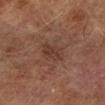Impression:
Recorded during total-body skin imaging; not selected for excision or biopsy.
Image and clinical context:
The subject is a male in their mid- to late 70s. Located on the left lower leg. The lesion's longest dimension is about 2.5 mm. This is a cross-polarized tile. Cropped from a total-body skin-imaging series; the visible field is about 15 mm.